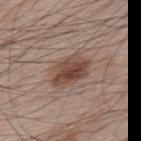| field | value |
|---|---|
| biopsy status | catalogued during a skin exam; not biopsied |
| anatomic site | the upper back |
| size | ~5 mm (longest diameter) |
| subject | male, in their mid-60s |
| tile lighting | white-light |
| imaging modality | 15 mm crop, total-body photography |